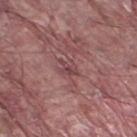Image and clinical context: The lesion's longest dimension is about 2.5 mm. The lesion is located on the left lower leg. A male subject aged around 40. The tile uses white-light illumination. This image is a 15 mm lesion crop taken from a total-body photograph.